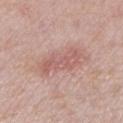Q: Was a biopsy performed?
A: catalogued during a skin exam; not biopsied
Q: Who is the patient?
A: female, aged approximately 60
Q: What is the imaging modality?
A: ~15 mm tile from a whole-body skin photo
Q: What is the anatomic site?
A: the left lower leg
Q: Illumination type?
A: white-light
Q: How large is the lesion?
A: about 6 mm